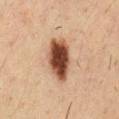biopsy_status: not biopsied; imaged during a skin examination
automated_metrics:
  cielab_L: 44
  cielab_a: 21
  cielab_b: 29
  vs_skin_contrast_norm: 14.0
  lesion_detection_confidence_0_100: 100
patient:
  sex: male
  age_approx: 35
site: chest
lesion_size:
  long_diameter_mm_approx: 5.5
lighting: cross-polarized
image:
  source: total-body photography crop
  field_of_view_mm: 15